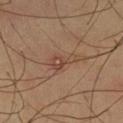{
  "biopsy_status": "not biopsied; imaged during a skin examination",
  "lighting": "cross-polarized",
  "patient": {
    "sex": "male",
    "age_approx": 60
  },
  "automated_metrics": {
    "area_mm2_approx": 4.5,
    "vs_skin_darker_L": 7.0,
    "vs_skin_contrast_norm": 6.0,
    "border_irregularity_0_10": 5.5,
    "color_variation_0_10": 2.5,
    "peripheral_color_asymmetry": 1.0,
    "nevus_likeness_0_100": 5
  },
  "image": {
    "source": "total-body photography crop",
    "field_of_view_mm": 15
  },
  "site": "left thigh",
  "lesion_size": {
    "long_diameter_mm_approx": 3.5
  }
}